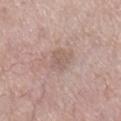Assessment: Part of a total-body skin-imaging series; this lesion was reviewed on a skin check and was not flagged for biopsy. Clinical summary: This is a white-light tile. A female subject, in their mid- to late 70s. On the right lower leg. A region of skin cropped from a whole-body photographic capture, roughly 15 mm wide. Measured at roughly 3 mm in maximum diameter. Automated tile analysis of the lesion measured a shape eccentricity near 0.45 and two-axis asymmetry of about 0.25. The software also gave a lesion color around L≈58 a*≈16 b*≈24 in CIELAB, about 7 CIELAB-L* units darker than the surrounding skin, and a lesion-to-skin contrast of about 4.5 (normalized; higher = more distinct). The software also gave a within-lesion color-variation index near 3/10 and peripheral color asymmetry of about 1.